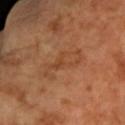Assessment:
No biopsy was performed on this lesion — it was imaged during a full skin examination and was not determined to be concerning.
Acquisition and patient details:
A 15 mm close-up extracted from a 3D total-body photography capture. On the right forearm. About 5 mm across. Automated tile analysis of the lesion measured a footprint of about 8 mm², an eccentricity of roughly 0.9, and a symmetry-axis asymmetry near 0.35. It also reported a color-variation rating of about 3/10 and radial color variation of about 1. The subject is a female aged around 70.